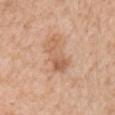notes: imaged on a skin check; not biopsied
subject: male, aged 58–62
body site: the right upper arm
lesion diameter: ≈5 mm
image: ~15 mm crop, total-body skin-cancer survey